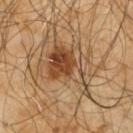{
  "biopsy_status": "not biopsied; imaged during a skin examination",
  "site": "upper back",
  "lighting": "cross-polarized",
  "image": {
    "source": "total-body photography crop",
    "field_of_view_mm": 15
  },
  "patient": {
    "sex": "male",
    "age_approx": 65
  },
  "lesion_size": {
    "long_diameter_mm_approx": 7.0
  }
}